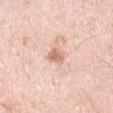Imaged during a routine full-body skin examination; the lesion was not biopsied and no histopathology is available. The total-body-photography lesion software estimated a lesion area of about 4 mm², an eccentricity of roughly 0.75, and two-axis asymmetry of about 0.3. The analysis additionally found a mean CIELAB color near L≈68 a*≈21 b*≈28 and a normalized border contrast of about 7. The software also gave a border-irregularity rating of about 2.5/10, a color-variation rating of about 1.5/10, and a peripheral color-asymmetry measure near 0.5. A region of skin cropped from a whole-body photographic capture, roughly 15 mm wide. Longest diameter approximately 3 mm. The lesion is on the right upper arm. A male patient approximately 35 years of age.